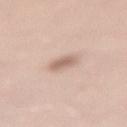This lesion was catalogued during total-body skin photography and was not selected for biopsy.
Located on the leg.
Captured under white-light illumination.
The subject is a male approximately 45 years of age.
This image is a 15 mm lesion crop taken from a total-body photograph.